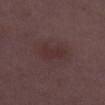Assessment:
This lesion was catalogued during total-body skin photography and was not selected for biopsy.
Context:
Imaged with white-light lighting. About 3 mm across. Cropped from a total-body skin-imaging series; the visible field is about 15 mm. A female subject aged around 30. From the right thigh.